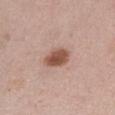<record>
<site>right thigh</site>
<patient>
  <sex>female</sex>
  <age_approx>40</age_approx>
</patient>
<image>
  <source>total-body photography crop</source>
  <field_of_view_mm>15</field_of_view_mm>
</image>
</record>